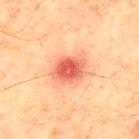| key | value |
|---|---|
| workup | imaged on a skin check; not biopsied |
| imaging modality | ~15 mm tile from a whole-body skin photo |
| patient | male, about 65 years old |
| lesion size | ~4 mm (longest diameter) |
| body site | the chest |